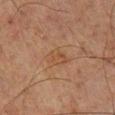biopsy status=imaged on a skin check; not biopsied
lesion diameter=about 3 mm
lighting=cross-polarized illumination
image source=15 mm crop, total-body photography
patient=male, about 65 years old
body site=the left lower leg